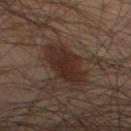Part of a total-body skin-imaging series; this lesion was reviewed on a skin check and was not flagged for biopsy.
From the leg.
A male patient approximately 55 years of age.
The tile uses cross-polarized illumination.
An algorithmic analysis of the crop reported a lesion area of about 15 mm², an outline eccentricity of about 0.85 (0 = round, 1 = elongated), and a symmetry-axis asymmetry near 0.2. The software also gave a lesion color around L≈21 a*≈13 b*≈18 in CIELAB, a lesion–skin lightness drop of about 7, and a normalized border contrast of about 8.5.
A 15 mm crop from a total-body photograph taken for skin-cancer surveillance.
Longest diameter approximately 6 mm.